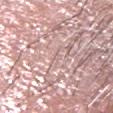Recorded during total-body skin imaging; not selected for excision or biopsy. A region of skin cropped from a whole-body photographic capture, roughly 15 mm wide. Approximately 1 mm at its widest. The lesion is on the head or neck. The lesion-visualizer software estimated a lesion area of about 0.5 mm², an outline eccentricity of about 0.8 (0 = round, 1 = elongated), and two-axis asymmetry of about 0.4. It also reported a lesion color around L≈65 a*≈20 b*≈20 in CIELAB. It also reported a border-irregularity index near 3/10, a within-lesion color-variation index near 0/10, and peripheral color asymmetry of about 0. It also reported a classifier nevus-likeness of about 0/100 and a detector confidence of about 0 out of 100 that the crop contains a lesion. A male subject in their mid- to late 80s or older. This is a white-light tile.